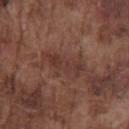notes: no biopsy performed (imaged during a skin exam) | subject: male, in their mid-70s | body site: the front of the torso | image source: ~15 mm crop, total-body skin-cancer survey | tile lighting: white-light illumination.